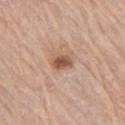No biopsy was performed on this lesion — it was imaged during a full skin examination and was not determined to be concerning. This is a white-light tile. A 15 mm crop from a total-body photograph taken for skin-cancer surveillance. A male subject, aged around 80. From the right upper arm.